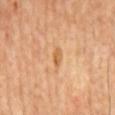Impression: No biopsy was performed on this lesion — it was imaged during a full skin examination and was not determined to be concerning. Background: The lesion's longest dimension is about 2.5 mm. An algorithmic analysis of the crop reported a mean CIELAB color near L≈60 a*≈23 b*≈42 and a lesion–skin lightness drop of about 9. It also reported border irregularity of about 3.5 on a 0–10 scale and a within-lesion color-variation index near 0/10. The software also gave a classifier nevus-likeness of about 0/100 and a detector confidence of about 100 out of 100 that the crop contains a lesion. The lesion is on the mid back. Captured under cross-polarized illumination. A male patient aged around 60. A region of skin cropped from a whole-body photographic capture, roughly 15 mm wide.